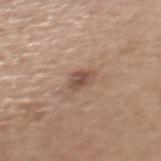Q: Is there a histopathology result?
A: imaged on a skin check; not biopsied
Q: Where on the body is the lesion?
A: the upper back
Q: How was this image acquired?
A: ~15 mm crop, total-body skin-cancer survey
Q: Automated lesion metrics?
A: a lesion area of about 4 mm² and two-axis asymmetry of about 0.25; a border-irregularity index near 2/10; an automated nevus-likeness rating near 40 out of 100
Q: Lesion size?
A: ≈2.5 mm
Q: What are the patient's age and sex?
A: female, in their mid-60s